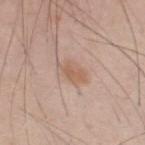Case summary:
– biopsy status — total-body-photography surveillance lesion; no biopsy
– TBP lesion metrics — a lesion area of about 7 mm² and a shape-asymmetry score of about 0.25 (0 = symmetric); a mean CIELAB color near L≈60 a*≈18 b*≈29, about 8 CIELAB-L* units darker than the surrounding skin, and a normalized border contrast of about 6; a border-irregularity rating of about 3/10 and a within-lesion color-variation index near 3.5/10; an automated nevus-likeness rating near 25 out of 100 and a detector confidence of about 100 out of 100 that the crop contains a lesion
– illumination — white-light illumination
– patient — male, aged 33–37
– imaging modality — 15 mm crop, total-body photography
– body site — the upper back